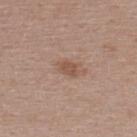Impression: The lesion was tiled from a total-body skin photograph and was not biopsied. Clinical summary: From the upper back. A female subject, aged approximately 45. A region of skin cropped from a whole-body photographic capture, roughly 15 mm wide.